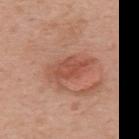lesion size = about 5.5 mm
patient = female, aged 48 to 52
image = ~15 mm tile from a whole-body skin photo
location = the upper back
illumination = white-light illumination